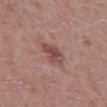<record>
<biopsy_status>not biopsied; imaged during a skin examination</biopsy_status>
<site>mid back</site>
<lighting>white-light</lighting>
<patient>
  <sex>male</sex>
  <age_approx>60</age_approx>
</patient>
<automated_metrics>
  <vs_skin_darker_L>10.0</vs_skin_darker_L>
  <color_variation_0_10>4.0</color_variation_0_10>
  <peripheral_color_asymmetry>1.5</peripheral_color_asymmetry>
  <nevus_likeness_0_100>5</nevus_likeness_0_100>
  <lesion_detection_confidence_0_100>100</lesion_detection_confidence_0_100>
</automated_metrics>
<image>
  <source>total-body photography crop</source>
  <field_of_view_mm>15</field_of_view_mm>
</image>
</record>